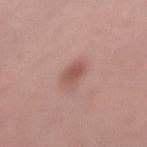notes=no biopsy performed (imaged during a skin exam) | image=15 mm crop, total-body photography | patient=female, in their mid-30s | body site=the left lower leg | lesion size=≈2.5 mm.